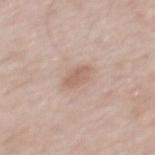Clinical impression: Part of a total-body skin-imaging series; this lesion was reviewed on a skin check and was not flagged for biopsy. Acquisition and patient details: Approximately 3 mm at its widest. From the back. Imaged with white-light lighting. A male patient, aged around 60. A 15 mm close-up tile from a total-body photography series done for melanoma screening. Automated image analysis of the tile measured a footprint of about 4.5 mm² and two-axis asymmetry of about 0.25. The software also gave roughly 8 lightness units darker than nearby skin and a normalized lesion–skin contrast near 6. And it measured a within-lesion color-variation index near 1.5/10.